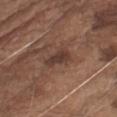Q: Is there a histopathology result?
A: imaged on a skin check; not biopsied
Q: Illumination type?
A: white-light illumination
Q: What is the imaging modality?
A: total-body-photography crop, ~15 mm field of view
Q: Who is the patient?
A: male, roughly 75 years of age
Q: Lesion size?
A: ≈4 mm
Q: What did automated image analysis measure?
A: a footprint of about 5.5 mm², a shape eccentricity near 0.9, and a symmetry-axis asymmetry near 0.3; an average lesion color of about L≈37 a*≈18 b*≈23 (CIELAB), roughly 9 lightness units darker than nearby skin, and a normalized border contrast of about 8; a border-irregularity index near 3.5/10, a within-lesion color-variation index near 2.5/10, and radial color variation of about 0.5; an automated nevus-likeness rating near 10 out of 100 and lesion-presence confidence of about 95/100
Q: Lesion location?
A: the arm